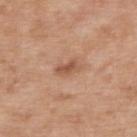Impression:
Part of a total-body skin-imaging series; this lesion was reviewed on a skin check and was not flagged for biopsy.
Clinical summary:
A lesion tile, about 15 mm wide, cut from a 3D total-body photograph. The lesion-visualizer software estimated an area of roughly 3.5 mm² and a shape-asymmetry score of about 0.25 (0 = symmetric). The analysis additionally found an average lesion color of about L≈54 a*≈22 b*≈32 (CIELAB) and a normalized border contrast of about 7. It also reported a border-irregularity rating of about 3/10, a color-variation rating of about 1.5/10, and a peripheral color-asymmetry measure near 0.5. And it measured a nevus-likeness score of about 5/100 and a detector confidence of about 100 out of 100 that the crop contains a lesion. On the upper back. The subject is a male aged around 50. This is a white-light tile.